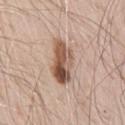Impression:
Captured during whole-body skin photography for melanoma surveillance; the lesion was not biopsied.
Background:
The patient is a male aged 68–72. A 15 mm crop from a total-body photograph taken for skin-cancer surveillance. The lesion is located on the right upper arm.